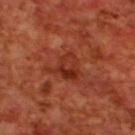Impression:
Recorded during total-body skin imaging; not selected for excision or biopsy.
Image and clinical context:
Cropped from a total-body skin-imaging series; the visible field is about 15 mm. The lesion is located on the back. The patient is a male aged 68–72.